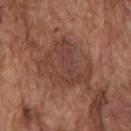Findings:
• biopsy status: imaged on a skin check; not biopsied
• location: the front of the torso
• imaging modality: 15 mm crop, total-body photography
• size: about 7 mm
• patient: male, aged approximately 75
• tile lighting: white-light illumination